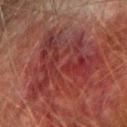Clinical impression: Imaged during a routine full-body skin examination; the lesion was not biopsied and no histopathology is available. Image and clinical context: A close-up tile cropped from a whole-body skin photograph, about 15 mm across. The patient is a male aged 73 to 77. Located on the arm.